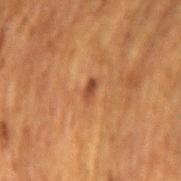follow-up: total-body-photography surveillance lesion; no biopsy
subject: female, aged 48–52
image source: total-body-photography crop, ~15 mm field of view
body site: the arm
tile lighting: cross-polarized